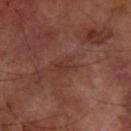workup=total-body-photography surveillance lesion; no biopsy | subject=male, about 65 years old | image source=~15 mm crop, total-body skin-cancer survey | site=the right forearm.